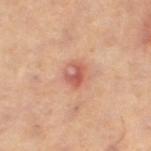Background: A female patient, in their 40s. Located on the left thigh. A 15 mm close-up extracted from a 3D total-body photography capture.